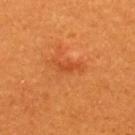Context: On the upper back. About 2.5 mm across. Automated tile analysis of the lesion measured a lesion area of about 2 mm², an eccentricity of roughly 0.9, and a symmetry-axis asymmetry near 0.4. It also reported a mean CIELAB color near L≈46 a*≈33 b*≈43 and a lesion-to-skin contrast of about 5.5 (normalized; higher = more distinct). The software also gave a border-irregularity index near 4.5/10 and a within-lesion color-variation index near 0/10. The analysis additionally found a nevus-likeness score of about 0/100 and a detector confidence of about 100 out of 100 that the crop contains a lesion. A female subject approximately 30 years of age. Cropped from a whole-body photographic skin survey; the tile spans about 15 mm. The tile uses cross-polarized illumination.